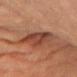workup: total-body-photography surveillance lesion; no biopsy | lesion diameter: ~5.5 mm (longest diameter) | illumination: cross-polarized illumination | subject: female, approximately 70 years of age | automated metrics: a mean CIELAB color near L≈36 a*≈22 b*≈26 and about 9 CIELAB-L* units darker than the surrounding skin | imaging modality: ~15 mm tile from a whole-body skin photo | site: the left upper arm.